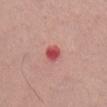workup: catalogued during a skin exam; not biopsied
site: the back
patient: male, aged 38 to 42
image: ~15 mm crop, total-body skin-cancer survey